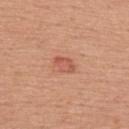Captured during whole-body skin photography for melanoma surveillance; the lesion was not biopsied.
Automated image analysis of the tile measured a border-irregularity index near 2/10, a color-variation rating of about 2.5/10, and a peripheral color-asymmetry measure near 1. It also reported a classifier nevus-likeness of about 65/100 and a lesion-detection confidence of about 100/100.
The tile uses white-light illumination.
Approximately 2.5 mm at its widest.
A lesion tile, about 15 mm wide, cut from a 3D total-body photograph.
On the upper back.
A male patient in their mid-50s.